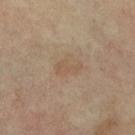{"automated_metrics": {"cielab_L": 54, "cielab_a": 15, "cielab_b": 30, "vs_skin_darker_L": 6.0, "vs_skin_contrast_norm": 4.5, "border_irregularity_0_10": 4.5, "color_variation_0_10": 2.0, "nevus_likeness_0_100": 5}, "image": {"source": "total-body photography crop", "field_of_view_mm": 15}, "lighting": "cross-polarized", "lesion_size": {"long_diameter_mm_approx": 3.0}, "site": "right lower leg", "patient": {"sex": "female", "age_approx": 65}}